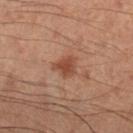Assessment: The lesion was tiled from a total-body skin photograph and was not biopsied. Image and clinical context: Measured at roughly 3 mm in maximum diameter. The tile uses cross-polarized illumination. The lesion is located on the right lower leg. A male subject aged around 50. A close-up tile cropped from a whole-body skin photograph, about 15 mm across.